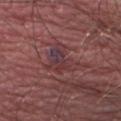Captured during whole-body skin photography for melanoma surveillance; the lesion was not biopsied. A male subject, aged approximately 40. On the abdomen. A roughly 15 mm field-of-view crop from a total-body skin photograph. Imaged with white-light lighting. Automated tile analysis of the lesion measured an area of roughly 10 mm² and two-axis asymmetry of about 0.5. The analysis additionally found a mean CIELAB color near L≈36 a*≈22 b*≈16, about 7 CIELAB-L* units darker than the surrounding skin, and a lesion-to-skin contrast of about 7 (normalized; higher = more distinct). The analysis additionally found a color-variation rating of about 9/10 and radial color variation of about 3.5.